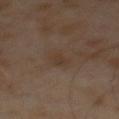The lesion was tiled from a total-body skin photograph and was not biopsied. Captured under cross-polarized illumination. Automated image analysis of the tile measured an area of roughly 3 mm², an outline eccentricity of about 0.85 (0 = round, 1 = elongated), and a shape-asymmetry score of about 0.25 (0 = symmetric). The analysis additionally found an average lesion color of about L≈34 a*≈13 b*≈24 (CIELAB), about 4 CIELAB-L* units darker than the surrounding skin, and a lesion-to-skin contrast of about 4.5 (normalized; higher = more distinct). The analysis additionally found a border-irregularity index near 2.5/10, a within-lesion color-variation index near 0.5/10, and radial color variation of about 0. It also reported a nevus-likeness score of about 0/100 and lesion-presence confidence of about 100/100. A male subject approximately 65 years of age. Cropped from a total-body skin-imaging series; the visible field is about 15 mm.